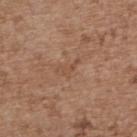Q: Was this lesion biopsied?
A: catalogued during a skin exam; not biopsied
Q: What are the patient's age and sex?
A: female, in their mid- to late 60s
Q: Lesion size?
A: ≈3 mm
Q: Lesion location?
A: the upper back
Q: What is the imaging modality?
A: total-body-photography crop, ~15 mm field of view
Q: Illumination type?
A: white-light